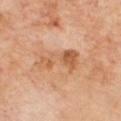Assessment:
This lesion was catalogued during total-body skin photography and was not selected for biopsy.
Context:
Cropped from a whole-body photographic skin survey; the tile spans about 15 mm. Captured under cross-polarized illumination. The subject is a male approximately 70 years of age. Located on the chest. Approximately 5 mm at its widest.